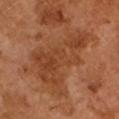Imaged during a routine full-body skin examination; the lesion was not biopsied and no histopathology is available. A male patient about 65 years old. A region of skin cropped from a whole-body photographic capture, roughly 15 mm wide. The lesion's longest dimension is about 8 mm. The tile uses cross-polarized illumination.